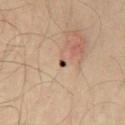Imaged during a routine full-body skin examination; the lesion was not biopsied and no histopathology is available.
On the chest.
A roughly 15 mm field-of-view crop from a total-body skin photograph.
Imaged with cross-polarized lighting.
A male subject about 50 years old.